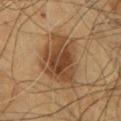Q: Is there a histopathology result?
A: no biopsy performed (imaged during a skin exam)
Q: What lighting was used for the tile?
A: cross-polarized
Q: Patient demographics?
A: male, aged 53 to 57
Q: What did automated image analysis measure?
A: a footprint of about 25 mm², an eccentricity of roughly 0.75, and a symmetry-axis asymmetry near 0.3; a lesion color around L≈41 a*≈17 b*≈30 in CIELAB; border irregularity of about 3.5 on a 0–10 scale, internal color variation of about 5.5 on a 0–10 scale, and peripheral color asymmetry of about 1.5; an automated nevus-likeness rating near 95 out of 100
Q: What is the anatomic site?
A: the front of the torso
Q: What is the lesion's diameter?
A: about 7 mm
Q: How was this image acquired?
A: 15 mm crop, total-body photography